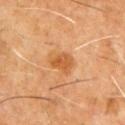Clinical impression: Recorded during total-body skin imaging; not selected for excision or biopsy. Clinical summary: Located on the chest. A male subject, aged 58 to 62. Cropped from a total-body skin-imaging series; the visible field is about 15 mm.